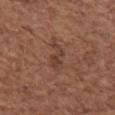Impression:
Captured during whole-body skin photography for melanoma surveillance; the lesion was not biopsied.
Acquisition and patient details:
A lesion tile, about 15 mm wide, cut from a 3D total-body photograph. About 3.5 mm across. The tile uses white-light illumination. The lesion is located on the arm. A female patient approximately 50 years of age.